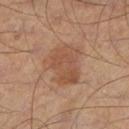workup = total-body-photography surveillance lesion; no biopsy
diameter = ~5 mm (longest diameter)
patient = male, roughly 55 years of age
lighting = cross-polarized illumination
anatomic site = the left lower leg
automated metrics = an automated nevus-likeness rating near 20 out of 100 and a lesion-detection confidence of about 100/100
imaging modality = 15 mm crop, total-body photography